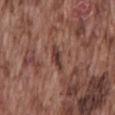biopsy status: no biopsy performed (imaged during a skin exam) | image source: ~15 mm tile from a whole-body skin photo | body site: the mid back | illumination: white-light illumination | automated metrics: a symmetry-axis asymmetry near 0.25; an average lesion color of about L≈38 a*≈21 b*≈23 (CIELAB); border irregularity of about 3.5 on a 0–10 scale, a within-lesion color-variation index near 1.5/10, and a peripheral color-asymmetry measure near 0.5 | lesion size: ≈3 mm | patient: male, approximately 75 years of age.